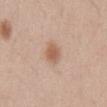No biopsy was performed on this lesion — it was imaged during a full skin examination and was not determined to be concerning. This is a white-light tile. Approximately 3 mm at its widest. A female subject, aged 33–37. From the abdomen. Automated tile analysis of the lesion measured an area of roughly 5.5 mm², an eccentricity of roughly 0.75, and a shape-asymmetry score of about 0.15 (0 = symmetric). The analysis additionally found a border-irregularity index near 1.5/10 and a color-variation rating of about 3.5/10. Cropped from a total-body skin-imaging series; the visible field is about 15 mm.